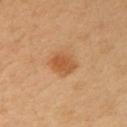Q: Was this lesion biopsied?
A: total-body-photography surveillance lesion; no biopsy
Q: What kind of image is this?
A: ~15 mm tile from a whole-body skin photo
Q: Lesion location?
A: the right upper arm
Q: How large is the lesion?
A: ≈3.5 mm
Q: What are the patient's age and sex?
A: male, in their 40s